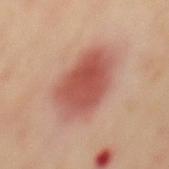Q: Was a biopsy performed?
A: imaged on a skin check; not biopsied
Q: How was this image acquired?
A: 15 mm crop, total-body photography
Q: Where on the body is the lesion?
A: the mid back
Q: Lesion size?
A: ≈7.5 mm
Q: What are the patient's age and sex?
A: male, in their 50s
Q: How was the tile lit?
A: cross-polarized illumination